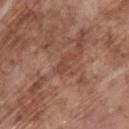Assessment:
The lesion was photographed on a routine skin check and not biopsied; there is no pathology result.
Acquisition and patient details:
A male patient approximately 70 years of age. About 3 mm across. This is a white-light tile. The lesion is located on the upper back. This image is a 15 mm lesion crop taken from a total-body photograph.